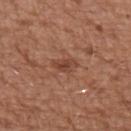follow-up=no biopsy performed (imaged during a skin exam)
imaging modality=~15 mm tile from a whole-body skin photo
patient=male, aged 73 to 77
lighting=white-light
site=the left upper arm